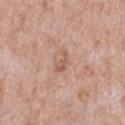Imaged during a routine full-body skin examination; the lesion was not biopsied and no histopathology is available.
The lesion is on the chest.
A roughly 15 mm field-of-view crop from a total-body skin photograph.
Captured under white-light illumination.
The lesion's longest dimension is about 3 mm.
The patient is a male approximately 60 years of age.
The total-body-photography lesion software estimated an average lesion color of about L≈58 a*≈21 b*≈29 (CIELAB) and a lesion–skin lightness drop of about 8. The software also gave a border-irregularity rating of about 6/10, a within-lesion color-variation index near 0.5/10, and a peripheral color-asymmetry measure near 0.